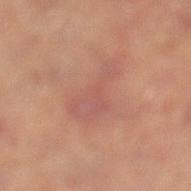{
  "biopsy_status": "not biopsied; imaged during a skin examination",
  "site": "left lower leg",
  "lesion_size": {
    "long_diameter_mm_approx": 5.5
  },
  "image": {
    "source": "total-body photography crop",
    "field_of_view_mm": 15
  },
  "patient": {
    "sex": "male",
    "age_approx": 50
  },
  "lighting": "cross-polarized"
}